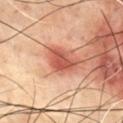The lesion was tiled from a total-body skin photograph and was not biopsied.
The lesion is located on the chest.
This is a cross-polarized tile.
A lesion tile, about 15 mm wide, cut from a 3D total-body photograph.
About 3 mm across.
The subject is a male about 70 years old.